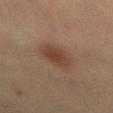This lesion was catalogued during total-body skin photography and was not selected for biopsy.
Captured under cross-polarized illumination.
The patient is a female approximately 65 years of age.
Measured at roughly 4.5 mm in maximum diameter.
Automated tile analysis of the lesion measured a footprint of about 9 mm² and two-axis asymmetry of about 0.2. And it measured an average lesion color of about L≈35 a*≈16 b*≈24 (CIELAB). The software also gave a color-variation rating of about 2/10.
From the mid back.
Cropped from a total-body skin-imaging series; the visible field is about 15 mm.